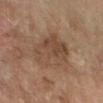The lesion was tiled from a total-body skin photograph and was not biopsied.
The lesion is located on the right forearm.
A roughly 15 mm field-of-view crop from a total-body skin photograph.
The lesion-visualizer software estimated about 8 CIELAB-L* units darker than the surrounding skin and a lesion-to-skin contrast of about 6.5 (normalized; higher = more distinct). The analysis additionally found a border-irregularity index near 3/10, internal color variation of about 5 on a 0–10 scale, and radial color variation of about 2. The analysis additionally found lesion-presence confidence of about 100/100.
A female subject, aged 58–62.